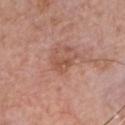Clinical impression: This lesion was catalogued during total-body skin photography and was not selected for biopsy. Acquisition and patient details: On the chest. The subject is a male in their mid-60s. The total-body-photography lesion software estimated a lesion area of about 5 mm², a shape eccentricity near 0.8, and two-axis asymmetry of about 0.5. The analysis additionally found border irregularity of about 5.5 on a 0–10 scale, a color-variation rating of about 2.5/10, and radial color variation of about 1. It also reported a classifier nevus-likeness of about 0/100. Approximately 3.5 mm at its widest. Cropped from a total-body skin-imaging series; the visible field is about 15 mm. Captured under white-light illumination.